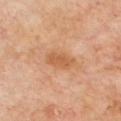Case summary:
– size · about 3.5 mm
– body site · the chest
– subject · male, approximately 70 years of age
– imaging modality · ~15 mm crop, total-body skin-cancer survey
– lighting · cross-polarized